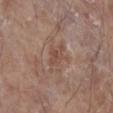Findings:
* notes — catalogued during a skin exam; not biopsied
* subject — male, aged 78 to 82
* automated metrics — an area of roughly 4 mm², an eccentricity of roughly 0.85, and a shape-asymmetry score of about 0.3 (0 = symmetric); a classifier nevus-likeness of about 0/100 and a lesion-detection confidence of about 100/100
* acquisition — ~15 mm crop, total-body skin-cancer survey
* location — the left lower leg
* lighting — white-light illumination